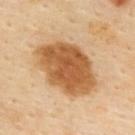{
  "biopsy_status": "not biopsied; imaged during a skin examination",
  "site": "back",
  "lighting": "cross-polarized",
  "image": {
    "source": "total-body photography crop",
    "field_of_view_mm": 15
  },
  "lesion_size": {
    "long_diameter_mm_approx": 8.0
  },
  "patient": {
    "sex": "female",
    "age_approx": 40
  }
}